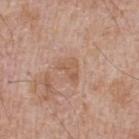Imaged during a routine full-body skin examination; the lesion was not biopsied and no histopathology is available.
The patient is a male aged approximately 65.
Measured at roughly 3.5 mm in maximum diameter.
This image is a 15 mm lesion crop taken from a total-body photograph.
This is a white-light tile.
From the upper back.
An algorithmic analysis of the crop reported a lesion color around L≈57 a*≈19 b*≈31 in CIELAB, a lesion–skin lightness drop of about 6, and a normalized lesion–skin contrast near 5. The software also gave an automated nevus-likeness rating near 0 out of 100.